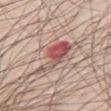Q: Was a biopsy performed?
A: total-body-photography surveillance lesion; no biopsy
Q: How large is the lesion?
A: ≈5.5 mm
Q: Where on the body is the lesion?
A: the mid back
Q: What kind of image is this?
A: total-body-photography crop, ~15 mm field of view
Q: Who is the patient?
A: male, in their 80s